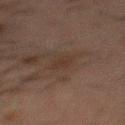biopsy status — imaged on a skin check; not biopsied | imaging modality — total-body-photography crop, ~15 mm field of view | illumination — cross-polarized illumination | subject — male, aged 48–52 | anatomic site — the back | TBP lesion metrics — an outline eccentricity of about 0.85 (0 = round, 1 = elongated) and a symmetry-axis asymmetry near 0.25; an average lesion color of about L≈25 a*≈12 b*≈19 (CIELAB), about 4 CIELAB-L* units darker than the surrounding skin, and a lesion-to-skin contrast of about 5 (normalized; higher = more distinct); border irregularity of about 3 on a 0–10 scale, internal color variation of about 0.5 on a 0–10 scale, and peripheral color asymmetry of about 0.